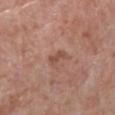This lesion was catalogued during total-body skin photography and was not selected for biopsy.
Located on the right lower leg.
A lesion tile, about 15 mm wide, cut from a 3D total-body photograph.
The recorded lesion diameter is about 2.5 mm.
An algorithmic analysis of the crop reported an automated nevus-likeness rating near 0 out of 100 and a detector confidence of about 100 out of 100 that the crop contains a lesion.
The patient is a male in their 70s.
The tile uses white-light illumination.